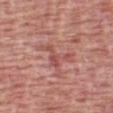This lesion was catalogued during total-body skin photography and was not selected for biopsy.
The lesion is on the chest.
A male patient, aged around 50.
A close-up tile cropped from a whole-body skin photograph, about 15 mm across.
The total-body-photography lesion software estimated a border-irregularity rating of about 9.5/10, a within-lesion color-variation index near 1/10, and a peripheral color-asymmetry measure near 0.5.
Approximately 5 mm at its widest.